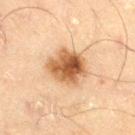Q: Was this lesion biopsied?
A: catalogued during a skin exam; not biopsied
Q: Lesion location?
A: the right thigh
Q: Automated lesion metrics?
A: a classifier nevus-likeness of about 95/100
Q: How large is the lesion?
A: about 4.5 mm
Q: Patient demographics?
A: male, in their 70s
Q: How was this image acquired?
A: ~15 mm crop, total-body skin-cancer survey
Q: What lighting was used for the tile?
A: cross-polarized